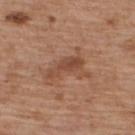This lesion was catalogued during total-body skin photography and was not selected for biopsy. The lesion is located on the upper back. The patient is a male in their mid-60s. The recorded lesion diameter is about 5 mm. A 15 mm close-up tile from a total-body photography series done for melanoma screening. Captured under white-light illumination.